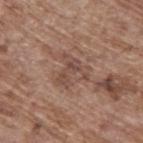Assessment:
Imaged during a routine full-body skin examination; the lesion was not biopsied and no histopathology is available.
Background:
Cropped from a total-body skin-imaging series; the visible field is about 15 mm. The lesion is located on the upper back. Measured at roughly 4 mm in maximum diameter. The tile uses white-light illumination. The patient is a male in their 70s. An algorithmic analysis of the crop reported an eccentricity of roughly 0.5 and two-axis asymmetry of about 0.65. The analysis additionally found a lesion color around L≈47 a*≈18 b*≈25 in CIELAB and roughly 7 lightness units darker than nearby skin.